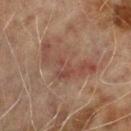This lesion was catalogued during total-body skin photography and was not selected for biopsy. On the right upper arm. A 15 mm close-up extracted from a 3D total-body photography capture. Measured at roughly 6.5 mm in maximum diameter. A male patient, about 70 years old. Automated tile analysis of the lesion measured an eccentricity of roughly 0.9 and a shape-asymmetry score of about 0.7 (0 = symmetric). The software also gave an average lesion color of about L≈37 a*≈18 b*≈22 (CIELAB), about 6 CIELAB-L* units darker than the surrounding skin, and a normalized lesion–skin contrast near 6. And it measured a border-irregularity rating of about 10/10, internal color variation of about 3 on a 0–10 scale, and a peripheral color-asymmetry measure near 1. This is a cross-polarized tile.